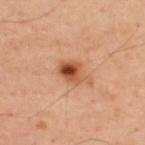Background:
Cropped from a total-body skin-imaging series; the visible field is about 15 mm. From the upper back. The total-body-photography lesion software estimated an area of roughly 6.5 mm², an outline eccentricity of about 0.75 (0 = round, 1 = elongated), and a shape-asymmetry score of about 0.3 (0 = symmetric). The analysis additionally found an automated nevus-likeness rating near 95 out of 100. This is a cross-polarized tile. A male subject in their mid-40s. About 4 mm across.